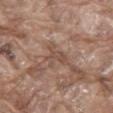| field | value |
|---|---|
| biopsy status | catalogued during a skin exam; not biopsied |
| location | the abdomen |
| patient | male, approximately 80 years of age |
| image | ~15 mm tile from a whole-body skin photo |